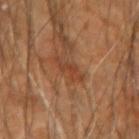This lesion was catalogued during total-body skin photography and was not selected for biopsy. The lesion-visualizer software estimated a footprint of about 4 mm², an outline eccentricity of about 0.9 (0 = round, 1 = elongated), and a symmetry-axis asymmetry near 0.45. And it measured an average lesion color of about L≈39 a*≈22 b*≈32 (CIELAB) and about 7 CIELAB-L* units darker than the surrounding skin. It also reported a border-irregularity rating of about 6.5/10, internal color variation of about 0 on a 0–10 scale, and a peripheral color-asymmetry measure near 0. From the left forearm. A 15 mm close-up tile from a total-body photography series done for melanoma screening. The patient is a male aged 58–62. Captured under cross-polarized illumination.